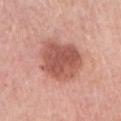biopsy status = catalogued during a skin exam; not biopsied | size = about 5.5 mm | illumination = white-light | automated lesion analysis = about 13 CIELAB-L* units darker than the surrounding skin and a normalized lesion–skin contrast near 8.5; a border-irregularity rating of about 2/10, a color-variation rating of about 4/10, and peripheral color asymmetry of about 1.5 | subject = female, aged around 65 | imaging modality = ~15 mm tile from a whole-body skin photo | anatomic site = the arm.